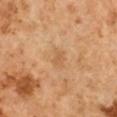Findings:
– workup · no biopsy performed (imaged during a skin exam)
– image · 15 mm crop, total-body photography
– size · about 2.5 mm
– anatomic site · the arm
– lighting · cross-polarized
– subject · male, aged approximately 60
– TBP lesion metrics · a mean CIELAB color near L≈56 a*≈20 b*≈38, about 6 CIELAB-L* units darker than the surrounding skin, and a normalized border contrast of about 4.5; a border-irregularity index near 3/10, a within-lesion color-variation index near 1.5/10, and radial color variation of about 0.5; a classifier nevus-likeness of about 0/100